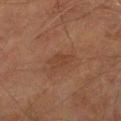Imaged during a routine full-body skin examination; the lesion was not biopsied and no histopathology is available. Captured under cross-polarized illumination. A 15 mm close-up tile from a total-body photography series done for melanoma screening. Approximately 3 mm at its widest. The subject is a male aged 73–77. From the right thigh.